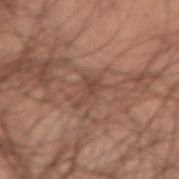Assessment: Captured during whole-body skin photography for melanoma surveillance; the lesion was not biopsied. Clinical summary: A roughly 15 mm field-of-view crop from a total-body skin photograph. On the right thigh. The patient is a male aged 43–47. Measured at roughly 3.5 mm in maximum diameter. Imaged with cross-polarized lighting.